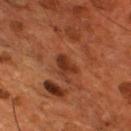| field | value |
|---|---|
| notes | catalogued during a skin exam; not biopsied |
| illumination | cross-polarized illumination |
| TBP lesion metrics | a mean CIELAB color near L≈31 a*≈25 b*≈31, a lesion–skin lightness drop of about 9, and a lesion-to-skin contrast of about 8.5 (normalized; higher = more distinct); a classifier nevus-likeness of about 5/100 |
| body site | the front of the torso |
| size | ~3 mm (longest diameter) |
| imaging modality | ~15 mm tile from a whole-body skin photo |
| patient | male, aged 53 to 57 |